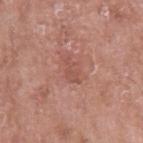biopsy_status: not biopsied; imaged during a skin examination
image:
  source: total-body photography crop
  field_of_view_mm: 15
lesion_size:
  long_diameter_mm_approx: 3.0
patient:
  sex: male
  age_approx: 55
site: arm
lighting: white-light